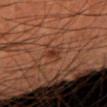Captured during whole-body skin photography for melanoma surveillance; the lesion was not biopsied. From the right forearm. The total-body-photography lesion software estimated an area of roughly 3.5 mm² and a shape eccentricity near 0.8. And it measured a border-irregularity index near 3.5/10, a within-lesion color-variation index near 2/10, and a peripheral color-asymmetry measure near 0.5. The software also gave a nevus-likeness score of about 5/100 and lesion-presence confidence of about 100/100. Measured at roughly 2.5 mm in maximum diameter. A 15 mm crop from a total-body photograph taken for skin-cancer surveillance. Captured under cross-polarized illumination. A male subject, aged approximately 60.